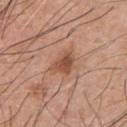Captured during whole-body skin photography for melanoma surveillance; the lesion was not biopsied.
A male patient, aged 58–62.
The total-body-photography lesion software estimated a mean CIELAB color near L≈51 a*≈23 b*≈31, roughly 11 lightness units darker than nearby skin, and a normalized lesion–skin contrast near 7.5. The software also gave a border-irregularity rating of about 3/10, internal color variation of about 4 on a 0–10 scale, and a peripheral color-asymmetry measure near 1.5. The software also gave a detector confidence of about 100 out of 100 that the crop contains a lesion.
Imaged with white-light lighting.
A 15 mm close-up tile from a total-body photography series done for melanoma screening.
The lesion's longest dimension is about 3.5 mm.
The lesion is on the chest.